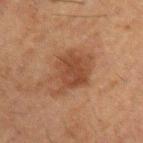Clinical impression: Imaged during a routine full-body skin examination; the lesion was not biopsied and no histopathology is available. Clinical summary: The tile uses cross-polarized illumination. Cropped from a whole-body photographic skin survey; the tile spans about 15 mm. A male patient, in their 50s. Located on the left upper arm. The lesion's longest dimension is about 5.5 mm.